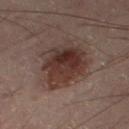workup: catalogued during a skin exam; not biopsied
anatomic site: the right thigh
illumination: cross-polarized
lesion diameter: about 6.5 mm
image-analysis metrics: a footprint of about 20 mm², an eccentricity of roughly 0.8, and a shape-asymmetry score of about 0.25 (0 = symmetric); a lesion-detection confidence of about 100/100
image: ~15 mm tile from a whole-body skin photo
patient: male, roughly 50 years of age